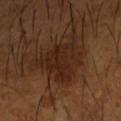workup = imaged on a skin check; not biopsied
patient = male, aged around 65
lighting = cross-polarized illumination
body site = the right forearm
acquisition = ~15 mm crop, total-body skin-cancer survey
size = ≈6 mm